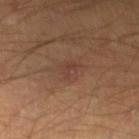{"biopsy_status": "not biopsied; imaged during a skin examination", "patient": {"sex": "male", "age_approx": 60}, "site": "left lower leg", "lighting": "cross-polarized", "lesion_size": {"long_diameter_mm_approx": 2.5}, "image": {"source": "total-body photography crop", "field_of_view_mm": 15}}